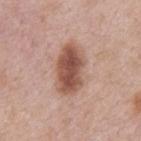{"biopsy_status": "not biopsied; imaged during a skin examination", "site": "upper back", "lighting": "white-light", "lesion_size": {"long_diameter_mm_approx": 6.0}, "patient": {"sex": "male", "age_approx": 55}, "image": {"source": "total-body photography crop", "field_of_view_mm": 15}}